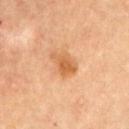Part of a total-body skin-imaging series; this lesion was reviewed on a skin check and was not flagged for biopsy. From the right upper arm. A male subject aged approximately 65. A 15 mm crop from a total-body photograph taken for skin-cancer surveillance.